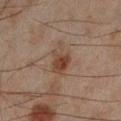A male patient, aged around 45. A 15 mm close-up tile from a total-body photography series done for melanoma screening. The recorded lesion diameter is about 4 mm. From the left lower leg. An algorithmic analysis of the crop reported a shape eccentricity near 0.75 and a shape-asymmetry score of about 0.3 (0 = symmetric). It also reported border irregularity of about 3.5 on a 0–10 scale, a color-variation rating of about 5.5/10, and radial color variation of about 2. The software also gave a classifier nevus-likeness of about 90/100. This is a cross-polarized tile.